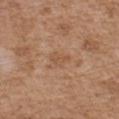| key | value |
|---|---|
| anatomic site | the chest |
| tile lighting | white-light |
| subject | male, aged approximately 75 |
| imaging modality | ~15 mm crop, total-body skin-cancer survey |